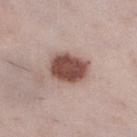Imaged during a routine full-body skin examination; the lesion was not biopsied and no histopathology is available. A 15 mm close-up extracted from a 3D total-body photography capture. Longest diameter approximately 4.5 mm. Imaged with white-light lighting. Located on the right lower leg. Automated image analysis of the tile measured a lesion color around L≈49 a*≈20 b*≈23 in CIELAB. And it measured border irregularity of about 1.5 on a 0–10 scale, internal color variation of about 3 on a 0–10 scale, and radial color variation of about 1. A female patient, aged around 30.